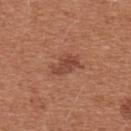Captured during whole-body skin photography for melanoma surveillance; the lesion was not biopsied. The lesion-visualizer software estimated a footprint of about 5.5 mm², an outline eccentricity of about 0.8 (0 = round, 1 = elongated), and a shape-asymmetry score of about 0.3 (0 = symmetric). The software also gave about 9 CIELAB-L* units darker than the surrounding skin and a lesion-to-skin contrast of about 7 (normalized; higher = more distinct). It also reported an automated nevus-likeness rating near 60 out of 100 and a lesion-detection confidence of about 100/100. Imaged with white-light lighting. The recorded lesion diameter is about 3.5 mm. A female patient aged 33 to 37. Cropped from a total-body skin-imaging series; the visible field is about 15 mm. The lesion is located on the front of the torso.